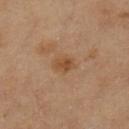follow-up=catalogued during a skin exam; not biopsied
subject=female, aged approximately 60
body site=the left lower leg
lighting=cross-polarized illumination
acquisition=15 mm crop, total-body photography